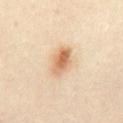– follow-up: imaged on a skin check; not biopsied
– body site: the abdomen
– patient: female, in their 40s
– acquisition: ~15 mm crop, total-body skin-cancer survey